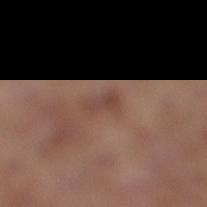Findings:
- biopsy status · imaged on a skin check; not biopsied
- location · the right lower leg
- image source · total-body-photography crop, ~15 mm field of view
- patient · male, approximately 80 years of age